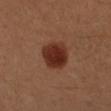Q: Was a biopsy performed?
A: catalogued during a skin exam; not biopsied
Q: What is the anatomic site?
A: the left forearm
Q: What did automated image analysis measure?
A: a border-irregularity index near 1.5/10 and a color-variation rating of about 3.5/10
Q: Patient demographics?
A: female, about 45 years old
Q: Illumination type?
A: cross-polarized illumination
Q: What kind of image is this?
A: total-body-photography crop, ~15 mm field of view